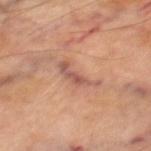Clinical impression: This lesion was catalogued during total-body skin photography and was not selected for biopsy. Image and clinical context: A region of skin cropped from a whole-body photographic capture, roughly 15 mm wide. Captured under cross-polarized illumination. On the right thigh. The subject is a male about 70 years old. Automated tile analysis of the lesion measured a within-lesion color-variation index near 2/10 and radial color variation of about 0.5. Longest diameter approximately 4.5 mm.